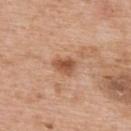Recorded during total-body skin imaging; not selected for excision or biopsy.
A region of skin cropped from a whole-body photographic capture, roughly 15 mm wide.
Automated image analysis of the tile measured a footprint of about 5 mm², an eccentricity of roughly 0.6, and a symmetry-axis asymmetry near 0.25.
The lesion is on the upper back.
This is a white-light tile.
A male subject about 60 years old.
The recorded lesion diameter is about 2.5 mm.